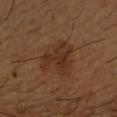No biopsy was performed on this lesion — it was imaged during a full skin examination and was not determined to be concerning. The lesion's longest dimension is about 4.5 mm. From the right forearm. Captured under cross-polarized illumination. A male patient, in their mid-60s. Cropped from a whole-body photographic skin survey; the tile spans about 15 mm.